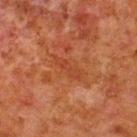{"biopsy_status": "not biopsied; imaged during a skin examination", "site": "back", "lesion_size": {"long_diameter_mm_approx": 3.5}, "image": {"source": "total-body photography crop", "field_of_view_mm": 15}, "patient": {"sex": "male", "age_approx": 80}}